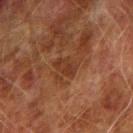{"biopsy_status": "not biopsied; imaged during a skin examination", "lighting": "cross-polarized", "patient": {"sex": "male", "age_approx": 75}, "automated_metrics": {"area_mm2_approx": 4.5, "eccentricity": 0.8, "cielab_L": 29, "cielab_a": 20, "cielab_b": 27, "vs_skin_darker_L": 6.0, "border_irregularity_0_10": 2.0, "color_variation_0_10": 1.5, "peripheral_color_asymmetry": 0.5}, "image": {"source": "total-body photography crop", "field_of_view_mm": 15}, "site": "arm", "lesion_size": {"long_diameter_mm_approx": 3.0}}